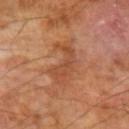biopsy status=no biopsy performed (imaged during a skin exam) | subject=male, aged 68–72 | anatomic site=the left forearm | acquisition=15 mm crop, total-body photography.